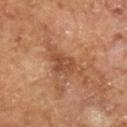  biopsy_status: not biopsied; imaged during a skin examination
  image:
    source: total-body photography crop
    field_of_view_mm: 15
  patient:
    sex: male
    age_approx: 65
  lighting: cross-polarized